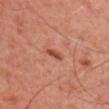| field | value |
|---|---|
| workup | total-body-photography surveillance lesion; no biopsy |
| image-analysis metrics | an area of roughly 2.5 mm², a shape eccentricity near 0.85, and a symmetry-axis asymmetry near 0.3; an average lesion color of about L≈46 a*≈27 b*≈31 (CIELAB), about 10 CIELAB-L* units darker than the surrounding skin, and a normalized lesion–skin contrast near 8; border irregularity of about 3 on a 0–10 scale, internal color variation of about 0.5 on a 0–10 scale, and radial color variation of about 0; a detector confidence of about 100 out of 100 that the crop contains a lesion |
| location | the right upper arm |
| image | ~15 mm tile from a whole-body skin photo |
| tile lighting | cross-polarized illumination |
| lesion diameter | ~2.5 mm (longest diameter) |
| patient | male, approximately 45 years of age |